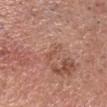Impression: Part of a total-body skin-imaging series; this lesion was reviewed on a skin check and was not flagged for biopsy. Background: This is a white-light tile. The total-body-photography lesion software estimated a lesion area of about 2 mm², an outline eccentricity of about 0.95 (0 = round, 1 = elongated), and two-axis asymmetry of about 0.45. The analysis additionally found an automated nevus-likeness rating near 0 out of 100 and a lesion-detection confidence of about 85/100. A male subject, in their 60s. Cropped from a total-body skin-imaging series; the visible field is about 15 mm. The lesion's longest dimension is about 3 mm. The lesion is located on the head or neck.